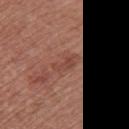Impression:
Imaged during a routine full-body skin examination; the lesion was not biopsied and no histopathology is available.
Background:
This image is a 15 mm lesion crop taken from a total-body photograph. A female subject, approximately 65 years of age. From the chest. About 3 mm across. Imaged with white-light lighting. The total-body-photography lesion software estimated a footprint of about 3 mm², an eccentricity of roughly 0.9, and a symmetry-axis asymmetry near 0.45. The analysis additionally found a border-irregularity rating of about 5/10, a color-variation rating of about 0/10, and peripheral color asymmetry of about 0.